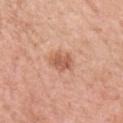workup = imaged on a skin check; not biopsied.